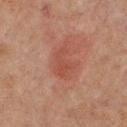follow-up: catalogued during a skin exam; not biopsied
illumination: cross-polarized illumination
subject: male, approximately 65 years of age
location: the upper back
lesion size: about 3.5 mm
image: total-body-photography crop, ~15 mm field of view